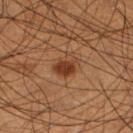notes: imaged on a skin check; not biopsied | subject: male, aged approximately 45 | anatomic site: the right lower leg | image source: total-body-photography crop, ~15 mm field of view | image-analysis metrics: a border-irregularity rating of about 3/10, internal color variation of about 4.5 on a 0–10 scale, and radial color variation of about 1.5; a nevus-likeness score of about 90/100 and lesion-presence confidence of about 100/100.